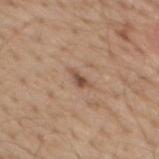This lesion was catalogued during total-body skin photography and was not selected for biopsy. A male patient, approximately 70 years of age. This image is a 15 mm lesion crop taken from a total-body photograph. Located on the chest.